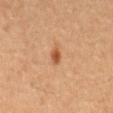| key | value |
|---|---|
| notes | total-body-photography surveillance lesion; no biopsy |
| acquisition | ~15 mm crop, total-body skin-cancer survey |
| lighting | cross-polarized illumination |
| automated lesion analysis | a footprint of about 3 mm², an outline eccentricity of about 0.8 (0 = round, 1 = elongated), and a symmetry-axis asymmetry near 0.2; a border-irregularity index near 2/10, internal color variation of about 2 on a 0–10 scale, and radial color variation of about 0.5; a nevus-likeness score of about 80/100 |
| subject | male, in their mid- to late 60s |
| anatomic site | the mid back |
| size | ~2.5 mm (longest diameter) |